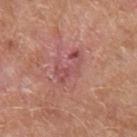This lesion was catalogued during total-body skin photography and was not selected for biopsy.
A male subject aged 63–67.
Captured under white-light illumination.
This image is a 15 mm lesion crop taken from a total-body photograph.
The lesion-visualizer software estimated a footprint of about 9.5 mm², an outline eccentricity of about 0.8 (0 = round, 1 = elongated), and a symmetry-axis asymmetry near 0.4. It also reported a border-irregularity index near 7/10, a color-variation rating of about 4.5/10, and a peripheral color-asymmetry measure near 1.5.
Longest diameter approximately 5 mm.
From the right upper arm.